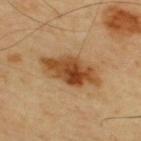Case summary:
* follow-up — imaged on a skin check; not biopsied
* automated lesion analysis — an outline eccentricity of about 0.9 (0 = round, 1 = elongated) and a symmetry-axis asymmetry near 0.25; a nevus-likeness score of about 90/100 and a detector confidence of about 100 out of 100 that the crop contains a lesion
* lighting — cross-polarized illumination
* anatomic site — the upper back
* patient — male, in their mid-60s
* acquisition — ~15 mm crop, total-body skin-cancer survey
* lesion size — ≈7 mm